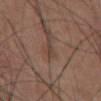Q: Was this lesion biopsied?
A: total-body-photography surveillance lesion; no biopsy
Q: What are the patient's age and sex?
A: male, roughly 55 years of age
Q: Lesion location?
A: the abdomen
Q: What kind of image is this?
A: ~15 mm crop, total-body skin-cancer survey